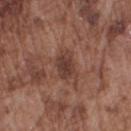Part of a total-body skin-imaging series; this lesion was reviewed on a skin check and was not flagged for biopsy. From the right upper arm. Measured at roughly 3 mm in maximum diameter. A close-up tile cropped from a whole-body skin photograph, about 15 mm across. The lesion-visualizer software estimated a footprint of about 6 mm² and an outline eccentricity of about 0.75 (0 = round, 1 = elongated). And it measured a border-irregularity rating of about 2.5/10 and radial color variation of about 1. The tile uses white-light illumination. A male patient, aged 73–77.